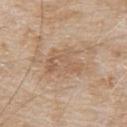| field | value |
|---|---|
| notes | catalogued during a skin exam; not biopsied |
| subject | male, in their 80s |
| acquisition | ~15 mm tile from a whole-body skin photo |
| anatomic site | the back |
| size | ≈5 mm |
| TBP lesion metrics | a lesion color around L≈58 a*≈17 b*≈31 in CIELAB, about 7 CIELAB-L* units darker than the surrounding skin, and a normalized border contrast of about 5 |